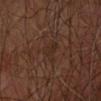| field | value |
|---|---|
| notes | imaged on a skin check; not biopsied |
| subject | male, in their mid- to late 60s |
| size | about 3 mm |
| site | the arm |
| imaging modality | total-body-photography crop, ~15 mm field of view |
| automated lesion analysis | an eccentricity of roughly 0.35 and a symmetry-axis asymmetry near 0.5; an average lesion color of about L≈25 a*≈15 b*≈22 (CIELAB), roughly 4 lightness units darker than nearby skin, and a lesion-to-skin contrast of about 5 (normalized; higher = more distinct); lesion-presence confidence of about 95/100 |